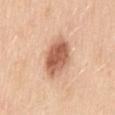follow-up: imaged on a skin check; not biopsied
TBP lesion metrics: an outline eccentricity of about 0.75 (0 = round, 1 = elongated); a lesion color around L≈61 a*≈23 b*≈33 in CIELAB and a normalized border contrast of about 9.5; border irregularity of about 1 on a 0–10 scale
diameter: about 5 mm
patient: female, aged 53–57
lighting: white-light
image source: ~15 mm crop, total-body skin-cancer survey
body site: the mid back